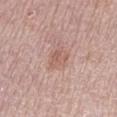Case summary:
• workup · catalogued during a skin exam; not biopsied
• acquisition · total-body-photography crop, ~15 mm field of view
• location · the leg
• lesion size · about 3 mm
• subject · female, approximately 65 years of age
• lighting · white-light